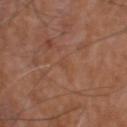follow-up: total-body-photography surveillance lesion; no biopsy
diameter: about 1.5 mm
subject: male, about 60 years old
anatomic site: the head or neck
imaging modality: 15 mm crop, total-body photography
automated metrics: a footprint of about 1.5 mm², a shape eccentricity near 0.65, and two-axis asymmetry of about 0.3; border irregularity of about 2.5 on a 0–10 scale, a color-variation rating of about 0/10, and radial color variation of about 0; lesion-presence confidence of about 85/100
illumination: white-light illumination